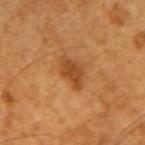– diameter: about 3.5 mm
– patient: male, aged around 60
– acquisition: ~15 mm crop, total-body skin-cancer survey
– illumination: cross-polarized
– anatomic site: the left forearm